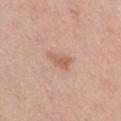Part of a total-body skin-imaging series; this lesion was reviewed on a skin check and was not flagged for biopsy. The tile uses white-light illumination. A 15 mm close-up tile from a total-body photography series done for melanoma screening. A female patient, about 45 years old. The lesion is located on the leg. The lesion's longest dimension is about 3 mm.